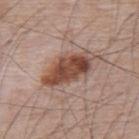Findings:
– biopsy status — catalogued during a skin exam; not biopsied
– lesion size — ≈6.5 mm
– illumination — white-light
– body site — the upper back
– subject — male, in their mid- to late 60s
– image source — total-body-photography crop, ~15 mm field of view